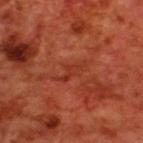The lesion was tiled from a total-body skin photograph and was not biopsied.
Cropped from a total-body skin-imaging series; the visible field is about 15 mm.
The lesion-visualizer software estimated an area of roughly 4 mm², a shape eccentricity near 0.9, and a symmetry-axis asymmetry near 0.55. It also reported a mean CIELAB color near L≈36 a*≈33 b*≈34, about 6 CIELAB-L* units darker than the surrounding skin, and a lesion-to-skin contrast of about 5 (normalized; higher = more distinct).
The lesion is located on the upper back.
Approximately 4 mm at its widest.
The tile uses cross-polarized illumination.
A male subject aged 68 to 72.